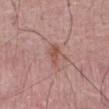follow-up: imaged on a skin check; not biopsied | automated lesion analysis: a footprint of about 4.5 mm², a shape eccentricity near 0.8, and two-axis asymmetry of about 0.3; border irregularity of about 3 on a 0–10 scale, a color-variation rating of about 2.5/10, and peripheral color asymmetry of about 1 | body site: the abdomen | image source: ~15 mm crop, total-body skin-cancer survey | patient: male, aged 48–52.